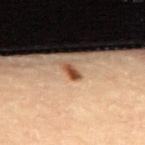Clinical impression: Recorded during total-body skin imaging; not selected for excision or biopsy. Context: This image is a 15 mm lesion crop taken from a total-body photograph. The lesion is on the upper back. Measured at roughly 2 mm in maximum diameter. A female patient, about 65 years old. Captured under cross-polarized illumination.